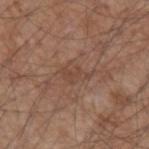Part of a total-body skin-imaging series; this lesion was reviewed on a skin check and was not flagged for biopsy.
The subject is a male aged 53 to 57.
From the left forearm.
A close-up tile cropped from a whole-body skin photograph, about 15 mm across.
Captured under white-light illumination.